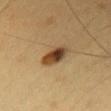patient = female, aged 28 to 32 | anatomic site = the head or neck | image source = total-body-photography crop, ~15 mm field of view.